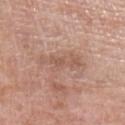notes=imaged on a skin check; not biopsied
patient=male, aged 78–82
lighting=white-light
lesion size=~5 mm (longest diameter)
image source=~15 mm tile from a whole-body skin photo
site=the leg
automated metrics=a within-lesion color-variation index near 2/10 and peripheral color asymmetry of about 0.5; lesion-presence confidence of about 100/100